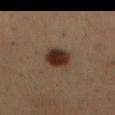Part of a total-body skin-imaging series; this lesion was reviewed on a skin check and was not flagged for biopsy.